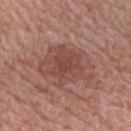• acquisition · ~15 mm crop, total-body skin-cancer survey
• location · the chest
• tile lighting · white-light
• patient · male, aged 73 to 77